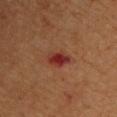{"biopsy_status": "not biopsied; imaged during a skin examination", "lesion_size": {"long_diameter_mm_approx": 3.0}, "image": {"source": "total-body photography crop", "field_of_view_mm": 15}, "site": "chest", "patient": {"sex": "male", "age_approx": 55}, "lighting": "cross-polarized"}